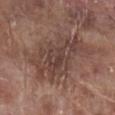Impression:
Imaged during a routine full-body skin examination; the lesion was not biopsied and no histopathology is available.
Acquisition and patient details:
The lesion is located on the right lower leg. The subject is a male approximately 70 years of age. A lesion tile, about 15 mm wide, cut from a 3D total-body photograph. Captured under white-light illumination. The total-body-photography lesion software estimated a lesion color around L≈42 a*≈18 b*≈22 in CIELAB, roughly 9 lightness units darker than nearby skin, and a normalized border contrast of about 7.5. It also reported a border-irregularity rating of about 5.5/10. And it measured a nevus-likeness score of about 0/100 and a detector confidence of about 85 out of 100 that the crop contains a lesion.